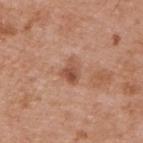{
  "biopsy_status": "not biopsied; imaged during a skin examination"
}